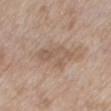Context: A female subject approximately 75 years of age. Imaged with white-light lighting. The recorded lesion diameter is about 5 mm. A close-up tile cropped from a whole-body skin photograph, about 15 mm across. Located on the mid back.